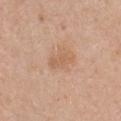| field | value |
|---|---|
| notes | total-body-photography surveillance lesion; no biopsy |
| automated metrics | a footprint of about 3.5 mm², an eccentricity of roughly 0.9, and a symmetry-axis asymmetry near 0.3; border irregularity of about 3.5 on a 0–10 scale and internal color variation of about 1 on a 0–10 scale |
| patient | female, aged approximately 40 |
| anatomic site | the front of the torso |
| image source | ~15 mm crop, total-body skin-cancer survey |
| diameter | ≈3 mm |
| lighting | white-light |